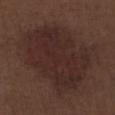follow-up: no biopsy performed (imaged during a skin exam) | patient: male, aged 68–72 | image: 15 mm crop, total-body photography | body site: the right lower leg.